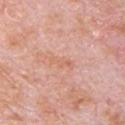notes=no biopsy performed (imaged during a skin exam); subject=male, aged 78 to 82; image=~15 mm crop, total-body skin-cancer survey; location=the chest.